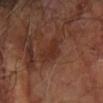Case summary:
* workup — imaged on a skin check; not biopsied
* lighting — cross-polarized illumination
* image-analysis metrics — radial color variation of about 0.5; a detector confidence of about 100 out of 100 that the crop contains a lesion
* subject — male, aged around 70
* size — about 3 mm
* image — ~15 mm tile from a whole-body skin photo
* site — the arm